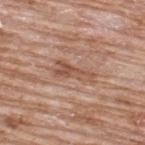Imaged with white-light lighting. The lesion-visualizer software estimated an area of roughly 7 mm². It also reported an automated nevus-likeness rating near 0 out of 100 and a detector confidence of about 60 out of 100 that the crop contains a lesion. The patient is a male about 65 years old. A 15 mm crop from a total-body photograph taken for skin-cancer surveillance. The recorded lesion diameter is about 4.5 mm. The lesion is on the back.